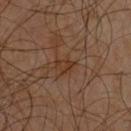subject = male, aged 58–62 | location = the chest | tile lighting = cross-polarized illumination | acquisition = total-body-photography crop, ~15 mm field of view | size = ≈2.5 mm.